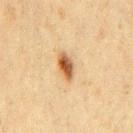Case summary:
– follow-up — no biopsy performed (imaged during a skin exam)
– image source — ~15 mm crop, total-body skin-cancer survey
– patient — male, aged approximately 60
– automated lesion analysis — about 15 CIELAB-L* units darker than the surrounding skin and a lesion-to-skin contrast of about 11 (normalized; higher = more distinct); a border-irregularity index near 1.5/10, a within-lesion color-variation index near 6.5/10, and peripheral color asymmetry of about 2; an automated nevus-likeness rating near 100 out of 100 and lesion-presence confidence of about 100/100
– anatomic site — the chest